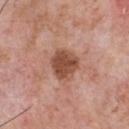The lesion was photographed on a routine skin check and not biopsied; there is no pathology result.
The subject is a male approximately 60 years of age.
Automated tile analysis of the lesion measured an average lesion color of about L≈49 a*≈23 b*≈30 (CIELAB), about 13 CIELAB-L* units darker than the surrounding skin, and a normalized lesion–skin contrast near 9.5. The software also gave internal color variation of about 3 on a 0–10 scale and a peripheral color-asymmetry measure near 1. The analysis additionally found an automated nevus-likeness rating near 20 out of 100.
Imaged with white-light lighting.
Longest diameter approximately 3.5 mm.
From the chest.
Cropped from a total-body skin-imaging series; the visible field is about 15 mm.